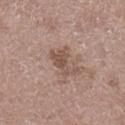image:
  source: total-body photography crop
  field_of_view_mm: 15
patient:
  sex: male
  age_approx: 50
lesion_size:
  long_diameter_mm_approx: 3.5
site: right lower leg